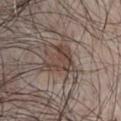Imaged during a routine full-body skin examination; the lesion was not biopsied and no histopathology is available. Automated image analysis of the tile measured a lesion color around L≈43 a*≈14 b*≈22 in CIELAB, a lesion–skin lightness drop of about 9, and a lesion-to-skin contrast of about 7.5 (normalized; higher = more distinct). And it measured an automated nevus-likeness rating near 40 out of 100 and a detector confidence of about 45 out of 100 that the crop contains a lesion. From the chest. About 4 mm across. Captured under white-light illumination. A region of skin cropped from a whole-body photographic capture, roughly 15 mm wide. The subject is a male approximately 55 years of age.